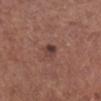Case summary:
- workup · imaged on a skin check; not biopsied
- TBP lesion metrics · an outline eccentricity of about 0.75 (0 = round, 1 = elongated) and two-axis asymmetry of about 0.25; a color-variation rating of about 5/10 and peripheral color asymmetry of about 2
- anatomic site · the right lower leg
- acquisition · ~15 mm crop, total-body skin-cancer survey
- subject · female, about 65 years old